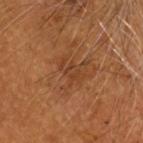notes — no biopsy performed (imaged during a skin exam)
subject — male, aged approximately 65
image — ~15 mm tile from a whole-body skin photo
site — the head or neck
diameter — ≈3 mm
lighting — cross-polarized
automated lesion analysis — a lesion area of about 4.5 mm², an eccentricity of roughly 0.85, and a shape-asymmetry score of about 0.35 (0 = symmetric); an automated nevus-likeness rating near 0 out of 100 and a detector confidence of about 100 out of 100 that the crop contains a lesion